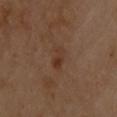Assessment:
Imaged during a routine full-body skin examination; the lesion was not biopsied and no histopathology is available.
Background:
Cropped from a total-body skin-imaging series; the visible field is about 15 mm. A male patient in their mid-50s. The lesion is on the upper back. An algorithmic analysis of the crop reported a shape eccentricity near 0.85 and a shape-asymmetry score of about 0.3 (0 = symmetric). And it measured a border-irregularity rating of about 3/10, a color-variation rating of about 1.5/10, and peripheral color asymmetry of about 0.